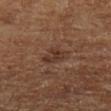The lesion was tiled from a total-body skin photograph and was not biopsied. The lesion is located on the left lower leg. A 15 mm close-up extracted from a 3D total-body photography capture. Longest diameter approximately 3 mm. A male patient, roughly 60 years of age. Imaged with cross-polarized lighting.